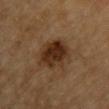This lesion was catalogued during total-body skin photography and was not selected for biopsy.
A male patient about 85 years old or older.
A 15 mm crop from a total-body photograph taken for skin-cancer surveillance.
The lesion is located on the chest.
The lesion's longest dimension is about 5 mm.